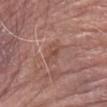Q: Was a biopsy performed?
A: no biopsy performed (imaged during a skin exam)
Q: What did automated image analysis measure?
A: roughly 7 lightness units darker than nearby skin; internal color variation of about 3.5 on a 0–10 scale and peripheral color asymmetry of about 1
Q: Where on the body is the lesion?
A: the left forearm
Q: What is the imaging modality?
A: 15 mm crop, total-body photography
Q: How was the tile lit?
A: white-light illumination
Q: Patient demographics?
A: male, roughly 80 years of age
Q: Lesion size?
A: ≈3 mm